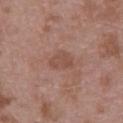{
  "patient": {
    "sex": "male",
    "age_approx": 55
  },
  "image": {
    "source": "total-body photography crop",
    "field_of_view_mm": 15
  },
  "automated_metrics": {
    "area_mm2_approx": 5.5,
    "eccentricity": 0.7,
    "shape_asymmetry": 0.25,
    "vs_skin_contrast_norm": 5.5
  },
  "lesion_size": {
    "long_diameter_mm_approx": 3.0
  },
  "site": "lower back"
}